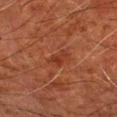No biopsy was performed on this lesion — it was imaged during a full skin examination and was not determined to be concerning. Imaged with cross-polarized lighting. A male subject about 80 years old. A close-up tile cropped from a whole-body skin photograph, about 15 mm across. The lesion-visualizer software estimated a border-irregularity rating of about 5/10, a within-lesion color-variation index near 0.5/10, and a peripheral color-asymmetry measure near 0. And it measured a nevus-likeness score of about 0/100 and a detector confidence of about 100 out of 100 that the crop contains a lesion. From the left upper arm.